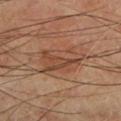biopsy status: imaged on a skin check; not biopsied | image-analysis metrics: an average lesion color of about L≈44 a*≈21 b*≈29 (CIELAB), roughly 8 lightness units darker than nearby skin, and a lesion-to-skin contrast of about 6 (normalized; higher = more distinct) | location: the leg | image source: ~15 mm tile from a whole-body skin photo | patient: male, aged 68–72.